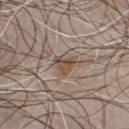Q: Was a biopsy performed?
A: no biopsy performed (imaged during a skin exam)
Q: What is the anatomic site?
A: the chest
Q: Patient demographics?
A: male, aged around 50
Q: Automated lesion metrics?
A: a border-irregularity index near 4/10; a nevus-likeness score of about 0/100 and a detector confidence of about 70 out of 100 that the crop contains a lesion
Q: How was this image acquired?
A: total-body-photography crop, ~15 mm field of view
Q: Illumination type?
A: white-light
Q: What is the lesion's diameter?
A: ≈3 mm